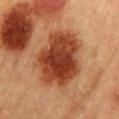Clinical impression: No biopsy was performed on this lesion — it was imaged during a full skin examination and was not determined to be concerning. Context: About 7.5 mm across. The subject is a female in their 60s. Located on the back. A region of skin cropped from a whole-body photographic capture, roughly 15 mm wide. This is a cross-polarized tile.